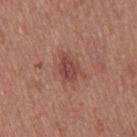notes=imaged on a skin check; not biopsied | lesion diameter=about 3.5 mm | patient=female, approximately 40 years of age | lighting=white-light | image=15 mm crop, total-body photography | site=the right thigh.